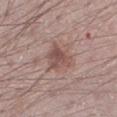Recorded during total-body skin imaging; not selected for excision or biopsy.
The tile uses white-light illumination.
A male patient aged 38–42.
Approximately 3.5 mm at its widest.
A lesion tile, about 15 mm wide, cut from a 3D total-body photograph.
Located on the leg.
Automated image analysis of the tile measured a lesion color around L≈51 a*≈19 b*≈22 in CIELAB, roughly 9 lightness units darker than nearby skin, and a lesion-to-skin contrast of about 7 (normalized; higher = more distinct).